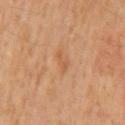follow-up: no biopsy performed (imaged during a skin exam)
imaging modality: ~15 mm crop, total-body skin-cancer survey
lesion diameter: ~2.5 mm (longest diameter)
location: the mid back
patient: male, aged approximately 60
tile lighting: cross-polarized illumination
image-analysis metrics: a footprint of about 3 mm², an outline eccentricity of about 0.85 (0 = round, 1 = elongated), and a symmetry-axis asymmetry near 0.4; an automated nevus-likeness rating near 0 out of 100 and a detector confidence of about 100 out of 100 that the crop contains a lesion